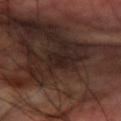| field | value |
|---|---|
| follow-up | no biopsy performed (imaged during a skin exam) |
| image-analysis metrics | a border-irregularity rating of about 4/10, a color-variation rating of about 2/10, and a peripheral color-asymmetry measure near 0.5; a nevus-likeness score of about 0/100 |
| illumination | cross-polarized |
| lesion size | ~2.5 mm (longest diameter) |
| image | ~15 mm tile from a whole-body skin photo |
| site | the right forearm |
| subject | male, approximately 60 years of age |